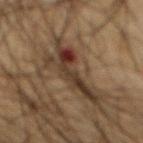Recorded during total-body skin imaging; not selected for excision or biopsy. Measured at roughly 7.5 mm in maximum diameter. A region of skin cropped from a whole-body photographic capture, roughly 15 mm wide. Located on the abdomen. Automated image analysis of the tile measured an average lesion color of about L≈28 a*≈13 b*≈22 (CIELAB) and about 10 CIELAB-L* units darker than the surrounding skin. The software also gave internal color variation of about 8 on a 0–10 scale and radial color variation of about 2.5. It also reported an automated nevus-likeness rating near 0 out of 100 and lesion-presence confidence of about 50/100. The patient is a male roughly 65 years of age. Captured under cross-polarized illumination.